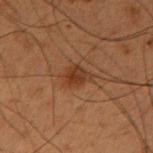Recorded during total-body skin imaging; not selected for excision or biopsy. A 15 mm close-up tile from a total-body photography series done for melanoma screening. A male patient, roughly 55 years of age. Located on the right upper arm.